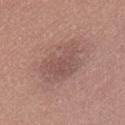Recorded during total-body skin imaging; not selected for excision or biopsy.